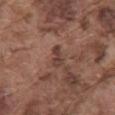Q: Patient demographics?
A: male, about 75 years old
Q: Illumination type?
A: white-light illumination
Q: Where on the body is the lesion?
A: the mid back
Q: How large is the lesion?
A: ≈2.5 mm
Q: Automated lesion metrics?
A: a lesion area of about 2.5 mm², an eccentricity of roughly 0.9, and a shape-asymmetry score of about 0.4 (0 = symmetric); an average lesion color of about L≈39 a*≈19 b*≈23 (CIELAB), roughly 9 lightness units darker than nearby skin, and a normalized lesion–skin contrast near 8; border irregularity of about 4 on a 0–10 scale, a color-variation rating of about 0/10, and radial color variation of about 0; a nevus-likeness score of about 10/100 and lesion-presence confidence of about 100/100
Q: What is the imaging modality?
A: ~15 mm tile from a whole-body skin photo